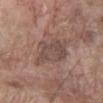Notes:
* notes — imaged on a skin check; not biopsied
* anatomic site — the arm
* patient — male, aged 58 to 62
* image — 15 mm crop, total-body photography